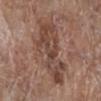Case summary:
• workup: no biopsy performed (imaged during a skin exam)
• acquisition: 15 mm crop, total-body photography
• lighting: white-light
• lesion diameter: ~9.5 mm (longest diameter)
• patient: female, aged around 80
• image-analysis metrics: a footprint of about 28 mm², an eccentricity of roughly 0.9, and a symmetry-axis asymmetry near 0.4; an average lesion color of about L≈45 a*≈19 b*≈25 (CIELAB) and a normalized lesion–skin contrast near 7; an automated nevus-likeness rating near 0 out of 100
• location: the right lower leg